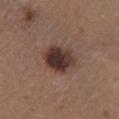<record>
  <biopsy_status>not biopsied; imaged during a skin examination</biopsy_status>
</record>